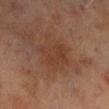Recorded during total-body skin imaging; not selected for excision or biopsy. The lesion is on the right lower leg. A male patient, in their 70s. Cropped from a whole-body photographic skin survey; the tile spans about 15 mm. Measured at roughly 4 mm in maximum diameter. Captured under cross-polarized illumination. The total-body-photography lesion software estimated a lesion color around L≈31 a*≈18 b*≈24 in CIELAB and a lesion–skin lightness drop of about 4. The software also gave an automated nevus-likeness rating near 0 out of 100.